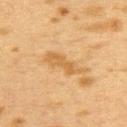Q: What is the anatomic site?
A: the upper back
Q: Automated lesion metrics?
A: a lesion area of about 7 mm², an outline eccentricity of about 0.95 (0 = round, 1 = elongated), and two-axis asymmetry of about 0.25; a lesion color around L≈51 a*≈17 b*≈38 in CIELAB
Q: How was this image acquired?
A: ~15 mm crop, total-body skin-cancer survey
Q: What lighting was used for the tile?
A: cross-polarized illumination
Q: Patient demographics?
A: female, aged approximately 40